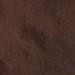This lesion was catalogued during total-body skin photography and was not selected for biopsy. Automated image analysis of the tile measured roughly 4 lightness units darker than nearby skin and a lesion-to-skin contrast of about 5.5 (normalized; higher = more distinct). It also reported a border-irregularity index near 5.5/10, a color-variation rating of about 1.5/10, and a peripheral color-asymmetry measure near 0.5. And it measured a nevus-likeness score of about 0/100 and lesion-presence confidence of about 75/100. Located on the right lower leg. A male patient, roughly 70 years of age. Approximately 5 mm at its widest. This image is a 15 mm lesion crop taken from a total-body photograph.